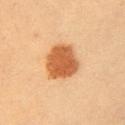Part of a total-body skin-imaging series; this lesion was reviewed on a skin check and was not flagged for biopsy. A female patient approximately 30 years of age. A region of skin cropped from a whole-body photographic capture, roughly 15 mm wide. The tile uses cross-polarized illumination. Longest diameter approximately 4.5 mm. The lesion is located on the left upper arm.